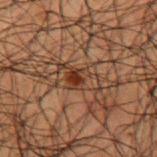{"biopsy_status": "not biopsied; imaged during a skin examination", "lighting": "cross-polarized", "patient": {"sex": "male", "age_approx": 50}, "image": {"source": "total-body photography crop", "field_of_view_mm": 15}, "lesion_size": {"long_diameter_mm_approx": 4.5}, "site": "left upper arm"}